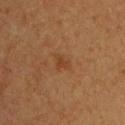Q: Is there a histopathology result?
A: imaged on a skin check; not biopsied
Q: What did automated image analysis measure?
A: an area of roughly 3 mm² and an eccentricity of roughly 0.75; a lesion color around L≈36 a*≈20 b*≈32 in CIELAB and a lesion-to-skin contrast of about 5.5 (normalized; higher = more distinct); a border-irregularity rating of about 4.5/10, internal color variation of about 0.5 on a 0–10 scale, and radial color variation of about 0
Q: How was this image acquired?
A: total-body-photography crop, ~15 mm field of view
Q: What are the patient's age and sex?
A: male, roughly 55 years of age
Q: Illumination type?
A: cross-polarized
Q: Lesion size?
A: ~2.5 mm (longest diameter)
Q: Lesion location?
A: the chest